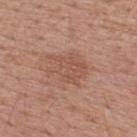workup: imaged on a skin check; not biopsied | illumination: white-light | lesion size: ≈5 mm | imaging modality: ~15 mm tile from a whole-body skin photo | patient: male, approximately 50 years of age | automated metrics: an area of roughly 11 mm², an eccentricity of roughly 0.65, and a symmetry-axis asymmetry near 0.35; a border-irregularity index near 5/10 and radial color variation of about 1 | body site: the upper back.